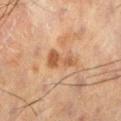<lesion>
<biopsy_status>not biopsied; imaged during a skin examination</biopsy_status>
<automated_metrics>
  <cielab_L>42</cielab_L>
  <cielab_a>18</cielab_a>
  <cielab_b>29</cielab_b>
  <vs_skin_darker_L>8.0</vs_skin_darker_L>
  <vs_skin_contrast_norm>7.0</vs_skin_contrast_norm>
</automated_metrics>
<patient>
  <sex>male</sex>
  <age_approx>60</age_approx>
</patient>
<lighting>cross-polarized</lighting>
<image>
  <source>total-body photography crop</source>
  <field_of_view_mm>15</field_of_view_mm>
</image>
<lesion_size>
  <long_diameter_mm_approx>4.5</long_diameter_mm_approx>
</lesion_size>
<site>left lower leg</site>
</lesion>